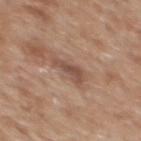Clinical impression: The lesion was photographed on a routine skin check and not biopsied; there is no pathology result. Context: A male subject about 60 years old. A region of skin cropped from a whole-body photographic capture, roughly 15 mm wide. The lesion is located on the back.